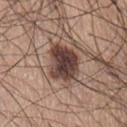This lesion was catalogued during total-body skin photography and was not selected for biopsy. On the front of the torso. Longest diameter approximately 4.5 mm. A lesion tile, about 15 mm wide, cut from a 3D total-body photograph. The patient is a female approximately 65 years of age. Automated tile analysis of the lesion measured an area of roughly 14 mm², a shape eccentricity near 0.6, and two-axis asymmetry of about 0.25. This is a white-light tile.